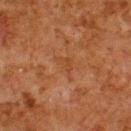Assessment:
Imaged during a routine full-body skin examination; the lesion was not biopsied and no histopathology is available.
Clinical summary:
On the upper back. A close-up tile cropped from a whole-body skin photograph, about 15 mm across. Captured under cross-polarized illumination. A male patient, about 80 years old. Approximately 3.5 mm at its widest.